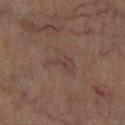Q: Is there a histopathology result?
A: catalogued during a skin exam; not biopsied
Q: Who is the patient?
A: in their mid-60s
Q: Lesion location?
A: the left lower leg
Q: What is the imaging modality?
A: total-body-photography crop, ~15 mm field of view